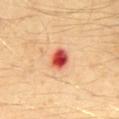The recorded lesion diameter is about 2.5 mm. A 15 mm close-up tile from a total-body photography series done for melanoma screening. A male patient, about 30 years old. The lesion-visualizer software estimated an area of roughly 5.5 mm² and a shape-asymmetry score of about 0.1 (0 = symmetric). The analysis additionally found a lesion color around L≈54 a*≈39 b*≈36 in CIELAB, roughly 20 lightness units darker than nearby skin, and a lesion-to-skin contrast of about 12.5 (normalized; higher = more distinct). The analysis additionally found a border-irregularity rating of about 1/10 and a within-lesion color-variation index near 9/10. The analysis additionally found lesion-presence confidence of about 100/100. Imaged with cross-polarized lighting. The lesion is on the mid back.